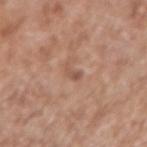This lesion was catalogued during total-body skin photography and was not selected for biopsy.
The lesion's longest dimension is about 2.5 mm.
Automated image analysis of the tile measured an area of roughly 2 mm² and two-axis asymmetry of about 0.55. It also reported a mean CIELAB color near L≈53 a*≈21 b*≈28, a lesion–skin lightness drop of about 9, and a normalized border contrast of about 6. It also reported a border-irregularity index near 5.5/10 and radial color variation of about 0. The analysis additionally found a classifier nevus-likeness of about 0/100 and a detector confidence of about 100 out of 100 that the crop contains a lesion.
From the arm.
A 15 mm crop from a total-body photograph taken for skin-cancer surveillance.
A male patient about 70 years old.